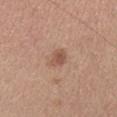workup — total-body-photography surveillance lesion; no biopsy
automated lesion analysis — an area of roughly 4 mm²; a border-irregularity index near 2.5/10, internal color variation of about 2.5 on a 0–10 scale, and a peripheral color-asymmetry measure near 1
image source — ~15 mm crop, total-body skin-cancer survey
site — the head or neck
subject — female, aged approximately 35
lesion size — ~3 mm (longest diameter)
illumination — white-light illumination